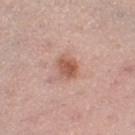The lesion was tiled from a total-body skin photograph and was not biopsied.
A 15 mm close-up extracted from a 3D total-body photography capture.
A female patient aged 33 to 37.
The lesion is on the left thigh.